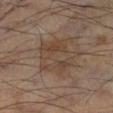Notes:
– notes: total-body-photography surveillance lesion; no biopsy
– patient: male, in their mid-50s
– image: 15 mm crop, total-body photography
– location: the right lower leg
– tile lighting: cross-polarized
– lesion diameter: about 5.5 mm